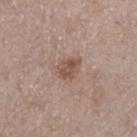Captured during whole-body skin photography for melanoma surveillance; the lesion was not biopsied.
The tile uses white-light illumination.
Approximately 3 mm at its widest.
A 15 mm close-up extracted from a 3D total-body photography capture.
A male patient in their mid-60s.
The lesion is on the left thigh.